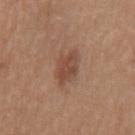Q: Is there a histopathology result?
A: catalogued during a skin exam; not biopsied
Q: Patient demographics?
A: male, aged 18–22
Q: Illumination type?
A: white-light
Q: What is the anatomic site?
A: the mid back
Q: What kind of image is this?
A: ~15 mm crop, total-body skin-cancer survey
Q: How large is the lesion?
A: about 3.5 mm